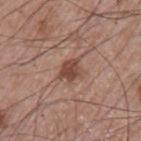No biopsy was performed on this lesion — it was imaged during a full skin examination and was not determined to be concerning.
The lesion is located on the left upper arm.
A male patient in their mid- to late 60s.
A 15 mm crop from a total-body photograph taken for skin-cancer surveillance.
This is a white-light tile.
An algorithmic analysis of the crop reported a footprint of about 5 mm² and a symmetry-axis asymmetry near 0.3. The software also gave a border-irregularity index near 3.5/10, internal color variation of about 2.5 on a 0–10 scale, and a peripheral color-asymmetry measure near 1. The analysis additionally found a nevus-likeness score of about 80/100 and a lesion-detection confidence of about 100/100.
About 3.5 mm across.